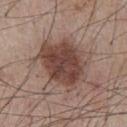The lesion was tiled from a total-body skin photograph and was not biopsied. The lesion is located on the chest. A male subject, approximately 55 years of age. This image is a 15 mm lesion crop taken from a total-body photograph.